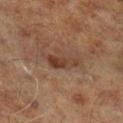| key | value |
|---|---|
| follow-up | total-body-photography surveillance lesion; no biopsy |
| lesion size | ~3.5 mm (longest diameter) |
| acquisition | ~15 mm tile from a whole-body skin photo |
| lighting | cross-polarized illumination |
| subject | male, aged approximately 70 |
| anatomic site | the right lower leg |